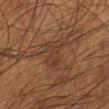{"biopsy_status": "not biopsied; imaged during a skin examination", "site": "left lower leg", "image": {"source": "total-body photography crop", "field_of_view_mm": 15}, "lighting": "cross-polarized", "patient": {"sex": "male", "age_approx": 55}}